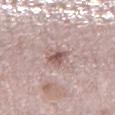notes: no biopsy performed (imaged during a skin exam)
patient: male, approximately 75 years of age
size: ≈2.5 mm
imaging modality: 15 mm crop, total-body photography
tile lighting: white-light
image-analysis metrics: a footprint of about 4 mm² and a shape eccentricity near 0.65; border irregularity of about 2.5 on a 0–10 scale and a color-variation rating of about 3.5/10; a nevus-likeness score of about 60/100 and a lesion-detection confidence of about 100/100
location: the leg